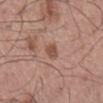Measured at roughly 3 mm in maximum diameter. A lesion tile, about 15 mm wide, cut from a 3D total-body photograph. The lesion is located on the back. A male patient aged around 55. Captured under white-light illumination.